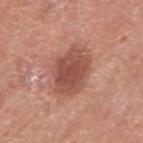{"biopsy_status": "not biopsied; imaged during a skin examination", "automated_metrics": {"cielab_L": 51, "cielab_a": 25, "cielab_b": 27, "border_irregularity_0_10": 2.5, "color_variation_0_10": 3.0, "peripheral_color_asymmetry": 1.0, "nevus_likeness_0_100": 65, "lesion_detection_confidence_0_100": 100}, "site": "right upper arm", "patient": {"sex": "male", "age_approx": 70}, "image": {"source": "total-body photography crop", "field_of_view_mm": 15}}